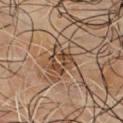Clinical summary: About 3.5 mm across. Imaged with cross-polarized lighting. A roughly 15 mm field-of-view crop from a total-body skin photograph. The patient is a male aged approximately 65. An algorithmic analysis of the crop reported an outline eccentricity of about 0.55 (0 = round, 1 = elongated) and a symmetry-axis asymmetry near 0.3. It also reported a border-irregularity index near 3.5/10, a color-variation rating of about 6.5/10, and radial color variation of about 2.5. The analysis additionally found a detector confidence of about 60 out of 100 that the crop contains a lesion. The lesion is located on the chest.